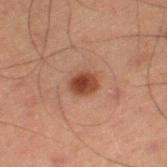Case summary:
• workup · no biopsy performed (imaged during a skin exam)
• lesion diameter · about 3 mm
• image source · 15 mm crop, total-body photography
• subject · male, aged 58–62
• lighting · cross-polarized
• location · the left thigh
• image-analysis metrics · a lesion area of about 5 mm² and two-axis asymmetry of about 0.25; a mean CIELAB color near L≈33 a*≈21 b*≈25 and about 11 CIELAB-L* units darker than the surrounding skin; a border-irregularity index near 2/10 and a within-lesion color-variation index near 3.5/10; an automated nevus-likeness rating near 100 out of 100 and a detector confidence of about 100 out of 100 that the crop contains a lesion